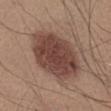Clinical impression: Recorded during total-body skin imaging; not selected for excision or biopsy. Background: The patient is a male in their mid-50s. Located on the abdomen. A close-up tile cropped from a whole-body skin photograph, about 15 mm across. The lesion's longest dimension is about 8 mm.